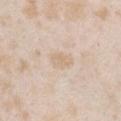Impression: No biopsy was performed on this lesion — it was imaged during a full skin examination and was not determined to be concerning. Acquisition and patient details: A female patient, aged 23 to 27. The total-body-photography lesion software estimated a border-irregularity rating of about 2.5/10 and peripheral color asymmetry of about 0.5. It also reported a classifier nevus-likeness of about 0/100 and lesion-presence confidence of about 100/100. The tile uses white-light illumination. Cropped from a whole-body photographic skin survey; the tile spans about 15 mm. The lesion is located on the chest.